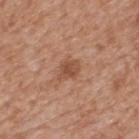follow-up = total-body-photography surveillance lesion; no biopsy | lighting = white-light illumination | image = 15 mm crop, total-body photography | image-analysis metrics = a lesion color around L≈50 a*≈23 b*≈32 in CIELAB and roughly 9 lightness units darker than nearby skin; a border-irregularity rating of about 2.5/10 and a peripheral color-asymmetry measure near 1; a lesion-detection confidence of about 100/100 | patient = male, aged 63–67 | size = ≈2.5 mm | body site = the mid back.